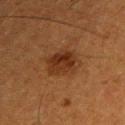biopsy status: catalogued during a skin exam; not biopsied
lesion diameter: about 4 mm
site: the right upper arm
acquisition: ~15 mm tile from a whole-body skin photo
TBP lesion metrics: an outline eccentricity of about 0.55 (0 = round, 1 = elongated) and a symmetry-axis asymmetry near 0.2; an average lesion color of about L≈26 a*≈18 b*≈27 (CIELAB), a lesion–skin lightness drop of about 7, and a normalized border contrast of about 8; an automated nevus-likeness rating near 70 out of 100 and lesion-presence confidence of about 100/100
subject: male, about 50 years old